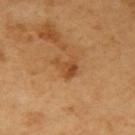Case summary:
• follow-up — total-body-photography surveillance lesion; no biopsy
• patient — male, approximately 65 years of age
• TBP lesion metrics — a mean CIELAB color near L≈48 a*≈23 b*≈40 and about 9 CIELAB-L* units darker than the surrounding skin
• illumination — cross-polarized
• acquisition — total-body-photography crop, ~15 mm field of view
• lesion diameter — ~3 mm (longest diameter)
• anatomic site — the arm